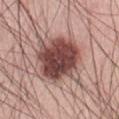Assessment:
This lesion was catalogued during total-body skin photography and was not selected for biopsy.
Clinical summary:
Captured under white-light illumination. The lesion's longest dimension is about 6 mm. A 15 mm close-up tile from a total-body photography series done for melanoma screening. The lesion is on the abdomen. The patient is a male roughly 60 years of age. An algorithmic analysis of the crop reported internal color variation of about 6.5 on a 0–10 scale.